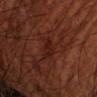The lesion was tiled from a total-body skin photograph and was not biopsied. The total-body-photography lesion software estimated a lesion color around L≈20 a*≈23 b*≈23 in CIELAB, roughly 6 lightness units darker than nearby skin, and a lesion-to-skin contrast of about 7 (normalized; higher = more distinct). The patient is a male in their 70s. On the left forearm. Cropped from a total-body skin-imaging series; the visible field is about 15 mm. This is a cross-polarized tile. Approximately 3.5 mm at its widest.